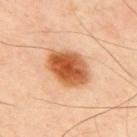The lesion was photographed on a routine skin check and not biopsied; there is no pathology result. Located on the upper back. Automated tile analysis of the lesion measured a footprint of about 16 mm² and an outline eccentricity of about 0.7 (0 = round, 1 = elongated). And it measured an average lesion color of about L≈57 a*≈27 b*≈41 (CIELAB) and a normalized lesion–skin contrast near 11.5. A male subject, aged 48–52. Imaged with cross-polarized lighting. A lesion tile, about 15 mm wide, cut from a 3D total-body photograph. The recorded lesion diameter is about 5.5 mm.